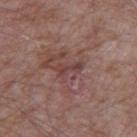| field | value |
|---|---|
| notes | total-body-photography surveillance lesion; no biopsy |